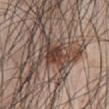{
  "lighting": "white-light",
  "patient": {
    "sex": "male",
    "age_approx": 45
  },
  "site": "abdomen",
  "lesion_size": {
    "long_diameter_mm_approx": 5.0
  },
  "automated_metrics": {
    "area_mm2_approx": 11.0,
    "eccentricity": 0.85,
    "shape_asymmetry": 0.45,
    "nevus_likeness_0_100": 0,
    "lesion_detection_confidence_0_100": 55
  },
  "image": {
    "source": "total-body photography crop",
    "field_of_view_mm": 15
  }
}